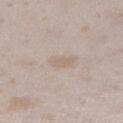Case summary:
– image — ~15 mm tile from a whole-body skin photo
– anatomic site — the right lower leg
– patient — female, in their mid-20s
– illumination — white-light
– size — ≈3 mm